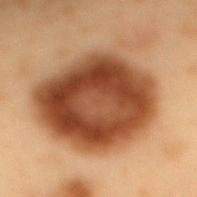biopsy status = no biopsy performed (imaged during a skin exam) | tile lighting = cross-polarized | body site = the mid back | automated metrics = a color-variation rating of about 8/10 and radial color variation of about 3; lesion-presence confidence of about 100/100 | subject = male, in their mid-50s | size = ~11.5 mm (longest diameter) | imaging modality = 15 mm crop, total-body photography.